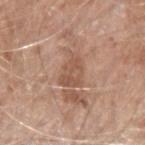Assessment:
This lesion was catalogued during total-body skin photography and was not selected for biopsy.
Image and clinical context:
Longest diameter approximately 3.5 mm. A 15 mm close-up extracted from a 3D total-body photography capture. The tile uses white-light illumination. On the right forearm. A male patient aged 58 to 62.